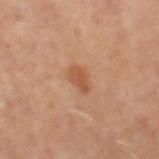Part of a total-body skin-imaging series; this lesion was reviewed on a skin check and was not flagged for biopsy. Located on the right thigh. Longest diameter approximately 3 mm. A 15 mm close-up extracted from a 3D total-body photography capture. The patient is a female approximately 50 years of age.